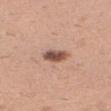Notes:
- follow-up: imaged on a skin check; not biopsied
- diameter: ≈3 mm
- automated metrics: an area of roughly 5 mm², an eccentricity of roughly 0.8, and two-axis asymmetry of about 0.25; a mean CIELAB color near L≈52 a*≈21 b*≈27 and about 16 CIELAB-L* units darker than the surrounding skin
- subject: male, approximately 40 years of age
- tile lighting: white-light
- site: the arm
- acquisition: 15 mm crop, total-body photography